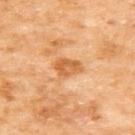biopsy_status: not biopsied; imaged during a skin examination
image:
  source: total-body photography crop
  field_of_view_mm: 15
patient:
  sex: female
  age_approx: 60
site: upper back